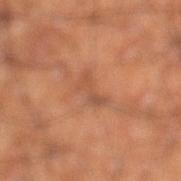biopsy status = total-body-photography surveillance lesion; no biopsy.